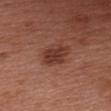lesion_size:
  long_diameter_mm_approx: 3.0
image:
  source: total-body photography crop
  field_of_view_mm: 15
automated_metrics:
  eccentricity: 0.7
  shape_asymmetry: 0.2
  cielab_L: 36
  cielab_a: 24
  cielab_b: 27
  vs_skin_darker_L: 10.0
  vs_skin_contrast_norm: 8.5
  border_irregularity_0_10: 2.0
  color_variation_0_10: 2.5
  peripheral_color_asymmetry: 1.0
  nevus_likeness_0_100: 90
  lesion_detection_confidence_0_100: 100
site: chest
patient:
  sex: female
  age_approx: 60
lighting: white-light